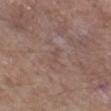Assessment: Captured during whole-body skin photography for melanoma surveillance; the lesion was not biopsied. Image and clinical context: A lesion tile, about 15 mm wide, cut from a 3D total-body photograph. The lesion is located on the left thigh. Imaged with white-light lighting. A male patient, roughly 65 years of age. The recorded lesion diameter is about 2 mm.